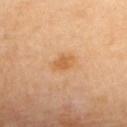Q: Was a biopsy performed?
A: catalogued during a skin exam; not biopsied
Q: Who is the patient?
A: female, in their 60s
Q: How was this image acquired?
A: 15 mm crop, total-body photography
Q: What is the anatomic site?
A: the back
Q: How was the tile lit?
A: cross-polarized